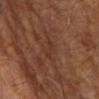A male subject aged 68–72.
Longest diameter approximately 3 mm.
Captured under cross-polarized illumination.
The lesion is on the left upper arm.
A close-up tile cropped from a whole-body skin photograph, about 15 mm across.